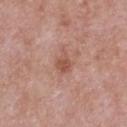Part of a total-body skin-imaging series; this lesion was reviewed on a skin check and was not flagged for biopsy. The recorded lesion diameter is about 3 mm. A region of skin cropped from a whole-body photographic capture, roughly 15 mm wide. From the chest. A male patient, in their mid-50s. Imaged with white-light lighting.